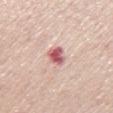Imaged during a routine full-body skin examination; the lesion was not biopsied and no histopathology is available. About 3 mm across. From the mid back. Cropped from a total-body skin-imaging series; the visible field is about 15 mm. Captured under white-light illumination. A male patient, aged 58–62.